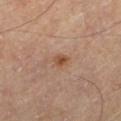notes = imaged on a skin check; not biopsied
tile lighting = cross-polarized
lesion diameter = ~2 mm (longest diameter)
subject = male, approximately 70 years of age
body site = the left thigh
acquisition = ~15 mm crop, total-body skin-cancer survey